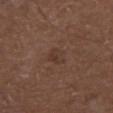  biopsy_status: not biopsied; imaged during a skin examination
  lighting: white-light
  patient:
    sex: male
    age_approx: 75
  automated_metrics:
    area_mm2_approx: 4.5
    eccentricity: 0.5
    shape_asymmetry: 0.3
    color_variation_0_10: 3.0
    peripheral_color_asymmetry: 1.0
  site: right lower leg
  image:
    source: total-body photography crop
    field_of_view_mm: 15